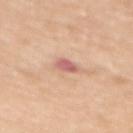Clinical impression: This lesion was catalogued during total-body skin photography and was not selected for biopsy. Image and clinical context: The patient is a female aged 53–57. The lesion is located on the mid back. This image is a 15 mm lesion crop taken from a total-body photograph. Imaged with white-light lighting. The recorded lesion diameter is about 2.5 mm.